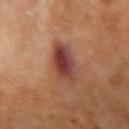Q: Was a biopsy performed?
A: no biopsy performed (imaged during a skin exam)
Q: Automated lesion metrics?
A: an area of roughly 10 mm², an eccentricity of roughly 0.7, and a shape-asymmetry score of about 0.2 (0 = symmetric)
Q: What kind of image is this?
A: ~15 mm tile from a whole-body skin photo
Q: Patient demographics?
A: female, aged approximately 70
Q: Where on the body is the lesion?
A: the arm